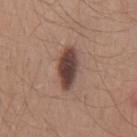The lesion was tiled from a total-body skin photograph and was not biopsied.